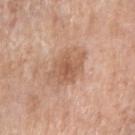Background: On the right upper arm. Cropped from a total-body skin-imaging series; the visible field is about 15 mm. A female patient, about 70 years old.